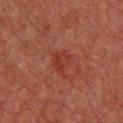<case>
  <biopsy_status>not biopsied; imaged during a skin examination</biopsy_status>
</case>